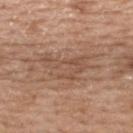No biopsy was performed on this lesion — it was imaged during a full skin examination and was not determined to be concerning.
A female patient about 60 years old.
On the upper back.
Cropped from a whole-body photographic skin survey; the tile spans about 15 mm.
The total-body-photography lesion software estimated an average lesion color of about L≈52 a*≈19 b*≈29 (CIELAB), about 8 CIELAB-L* units darker than the surrounding skin, and a lesion-to-skin contrast of about 5.5 (normalized; higher = more distinct). And it measured a classifier nevus-likeness of about 0/100 and a lesion-detection confidence of about 55/100.
The tile uses white-light illumination.
The recorded lesion diameter is about 6.5 mm.